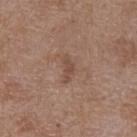The lesion was photographed on a routine skin check and not biopsied; there is no pathology result. A close-up tile cropped from a whole-body skin photograph, about 15 mm across. The lesion is on the upper back. This is a white-light tile. A female subject approximately 70 years of age. Measured at roughly 2.5 mm in maximum diameter.